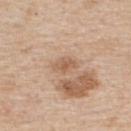Clinical impression:
Recorded during total-body skin imaging; not selected for excision or biopsy.
Clinical summary:
On the upper back. About 2.5 mm across. A male patient, in their 60s. A 15 mm close-up tile from a total-body photography series done for melanoma screening. Captured under white-light illumination.